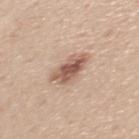Q: Is there a histopathology result?
A: imaged on a skin check; not biopsied
Q: How large is the lesion?
A: ≈4 mm
Q: How was this image acquired?
A: ~15 mm tile from a whole-body skin photo
Q: Where on the body is the lesion?
A: the front of the torso
Q: What are the patient's age and sex?
A: male, about 50 years old